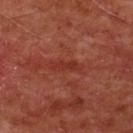Q: Was this lesion biopsied?
A: no biopsy performed (imaged during a skin exam)
Q: Illumination type?
A: cross-polarized
Q: What kind of image is this?
A: 15 mm crop, total-body photography
Q: What is the anatomic site?
A: the front of the torso
Q: Patient demographics?
A: male, aged 58 to 62